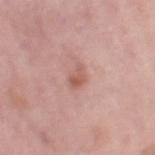Q: Is there a histopathology result?
A: imaged on a skin check; not biopsied
Q: How was the tile lit?
A: white-light
Q: What is the anatomic site?
A: the leg
Q: What is the imaging modality?
A: ~15 mm crop, total-body skin-cancer survey
Q: Who is the patient?
A: female, roughly 50 years of age
Q: How large is the lesion?
A: ~2.5 mm (longest diameter)